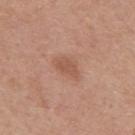Assessment:
Captured during whole-body skin photography for melanoma surveillance; the lesion was not biopsied.
Context:
The lesion-visualizer software estimated a footprint of about 4 mm², an eccentricity of roughly 0.8, and a symmetry-axis asymmetry near 0.25. The software also gave a mean CIELAB color near L≈54 a*≈23 b*≈30, roughly 8 lightness units darker than nearby skin, and a normalized border contrast of about 6. And it measured border irregularity of about 3 on a 0–10 scale. On the upper back. Longest diameter approximately 3 mm. A 15 mm close-up extracted from a 3D total-body photography capture. The patient is a male aged approximately 30. The tile uses white-light illumination.